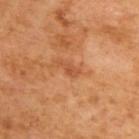Assessment: No biopsy was performed on this lesion — it was imaged during a full skin examination and was not determined to be concerning.